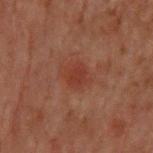| feature | finding |
|---|---|
| workup | imaged on a skin check; not biopsied |
| imaging modality | 15 mm crop, total-body photography |
| site | the back |
| subject | male, aged 68 to 72 |
| diameter | ~2.5 mm (longest diameter) |
| tile lighting | cross-polarized |